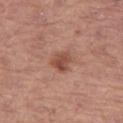Q: Is there a histopathology result?
A: no biopsy performed (imaged during a skin exam)
Q: What did automated image analysis measure?
A: a footprint of about 5 mm² and an outline eccentricity of about 0.5 (0 = round, 1 = elongated); a color-variation rating of about 3.5/10 and a peripheral color-asymmetry measure near 1
Q: Lesion size?
A: ≈2.5 mm
Q: How was this image acquired?
A: ~15 mm crop, total-body skin-cancer survey
Q: Where on the body is the lesion?
A: the left thigh
Q: Patient demographics?
A: male, in their mid- to late 60s